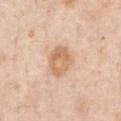A roughly 15 mm field-of-view crop from a total-body skin photograph.
A male subject aged around 50.
Located on the front of the torso.